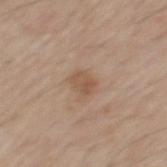Assessment: Captured during whole-body skin photography for melanoma surveillance; the lesion was not biopsied. Context: The lesion is on the mid back. A close-up tile cropped from a whole-body skin photograph, about 15 mm across. A male subject, aged 53 to 57.